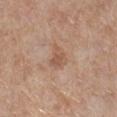This lesion was catalogued during total-body skin photography and was not selected for biopsy. From the right lower leg. A female patient about 45 years old. An algorithmic analysis of the crop reported border irregularity of about 3 on a 0–10 scale, a within-lesion color-variation index near 2.5/10, and radial color variation of about 1. Measured at roughly 2.5 mm in maximum diameter. A roughly 15 mm field-of-view crop from a total-body skin photograph.